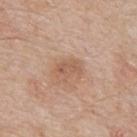Q: Was this lesion biopsied?
A: imaged on a skin check; not biopsied
Q: What is the anatomic site?
A: the back
Q: Automated lesion metrics?
A: a lesion color around L≈57 a*≈20 b*≈30 in CIELAB and a lesion–skin lightness drop of about 8; border irregularity of about 2.5 on a 0–10 scale and a within-lesion color-variation index near 3/10
Q: What kind of image is this?
A: ~15 mm crop, total-body skin-cancer survey
Q: Lesion size?
A: ≈3 mm
Q: Who is the patient?
A: male, in their mid- to late 60s
Q: Illumination type?
A: white-light illumination